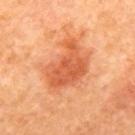Captured during whole-body skin photography for melanoma surveillance; the lesion was not biopsied.
A female subject, in their 50s.
A roughly 15 mm field-of-view crop from a total-body skin photograph.
Located on the upper back.
Longest diameter approximately 6 mm.